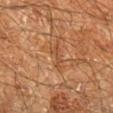Located on the right lower leg. The total-body-photography lesion software estimated a mean CIELAB color near L≈37 a*≈19 b*≈29 and a normalized border contrast of about 5.5. And it measured a border-irregularity rating of about 7/10, a within-lesion color-variation index near 0/10, and a peripheral color-asymmetry measure near 0. It also reported a nevus-likeness score of about 0/100 and lesion-presence confidence of about 65/100. A male patient in their 60s. Cropped from a total-body skin-imaging series; the visible field is about 15 mm.